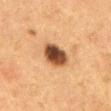The lesion was tiled from a total-body skin photograph and was not biopsied.
A female patient, aged around 50.
A 15 mm close-up tile from a total-body photography series done for melanoma screening.
Measured at roughly 4 mm in maximum diameter.
The lesion is on the abdomen.
This is a cross-polarized tile.